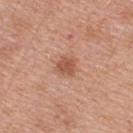follow-up: catalogued during a skin exam; not biopsied
image-analysis metrics: an area of roughly 5 mm² and an outline eccentricity of about 0.35 (0 = round, 1 = elongated)
patient: female, roughly 40 years of age
lighting: white-light illumination
image: ~15 mm tile from a whole-body skin photo
location: the upper back
size: ≈2.5 mm